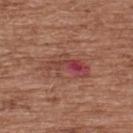Assessment: Imaged during a routine full-body skin examination; the lesion was not biopsied and no histopathology is available. Image and clinical context: This image is a 15 mm lesion crop taken from a total-body photograph. A male patient, aged around 75. The lesion is on the back.